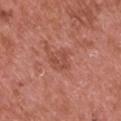| key | value |
|---|---|
| follow-up | total-body-photography surveillance lesion; no biopsy |